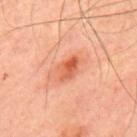This lesion was catalogued during total-body skin photography and was not selected for biopsy.
Approximately 3.5 mm at its widest.
The patient is a male about 50 years old.
A roughly 15 mm field-of-view crop from a total-body skin photograph.
On the back.
This is a cross-polarized tile.